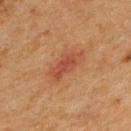  biopsy_status: not biopsied; imaged during a skin examination
  site: back
  lesion_size:
    long_diameter_mm_approx: 4.5
  lighting: cross-polarized
  image:
    source: total-body photography crop
    field_of_view_mm: 15
  patient:
    sex: male
    age_approx: 50